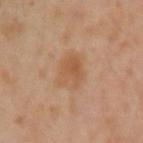TBP lesion metrics: a lesion area of about 12 mm², an eccentricity of roughly 0.4, and a symmetry-axis asymmetry near 0.2; border irregularity of about 2 on a 0–10 scale, a color-variation rating of about 4/10, and radial color variation of about 1.5 | size: ≈4 mm | patient: female, aged approximately 40 | acquisition: 15 mm crop, total-body photography | location: the left arm | tile lighting: cross-polarized illumination.